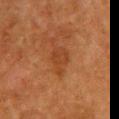Part of a total-body skin-imaging series; this lesion was reviewed on a skin check and was not flagged for biopsy. The lesion is on the chest. The subject is a female about 50 years old. A 15 mm crop from a total-body photograph taken for skin-cancer surveillance.